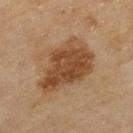notes=catalogued during a skin exam; not biopsied
location=the leg
patient=female, aged 58 to 62
lesion diameter=~7 mm (longest diameter)
acquisition=~15 mm tile from a whole-body skin photo